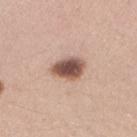No biopsy was performed on this lesion — it was imaged during a full skin examination and was not determined to be concerning. A female subject, about 25 years old. Cropped from a total-body skin-imaging series; the visible field is about 15 mm. The tile uses white-light illumination. The lesion's longest dimension is about 4 mm. The lesion is located on the right forearm.